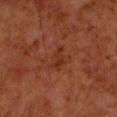This lesion was catalogued during total-body skin photography and was not selected for biopsy.
An algorithmic analysis of the crop reported a footprint of about 3.5 mm² and a shape-asymmetry score of about 0.35 (0 = symmetric). It also reported a mean CIELAB color near L≈30 a*≈25 b*≈31 and a lesion-to-skin contrast of about 6 (normalized; higher = more distinct). It also reported a detector confidence of about 100 out of 100 that the crop contains a lesion.
A male patient, aged 58–62.
This image is a 15 mm lesion crop taken from a total-body photograph.
The tile uses cross-polarized illumination.
From the left forearm.
Longest diameter approximately 3 mm.